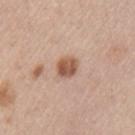No biopsy was performed on this lesion — it was imaged during a full skin examination and was not determined to be concerning. A lesion tile, about 15 mm wide, cut from a 3D total-body photograph. Longest diameter approximately 3 mm. This is a white-light tile. A male patient, aged 53–57. On the left upper arm.